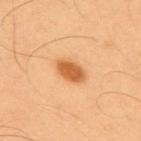Imaged during a routine full-body skin examination; the lesion was not biopsied and no histopathology is available.
A region of skin cropped from a whole-body photographic capture, roughly 15 mm wide.
Longest diameter approximately 4 mm.
Captured under cross-polarized illumination.
The lesion is located on the back.
A male patient aged 53 to 57.